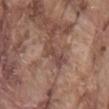  biopsy_status: not biopsied; imaged during a skin examination